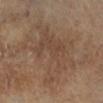Clinical impression:
No biopsy was performed on this lesion — it was imaged during a full skin examination and was not determined to be concerning.
Clinical summary:
A 15 mm crop from a total-body photograph taken for skin-cancer surveillance. A patient in their 60s. The lesion is on the right lower leg.